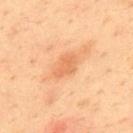image source — total-body-photography crop, ~15 mm field of view
image-analysis metrics — an average lesion color of about L≈61 a*≈26 b*≈39 (CIELAB), about 8 CIELAB-L* units darker than the surrounding skin, and a lesion-to-skin contrast of about 5.5 (normalized; higher = more distinct); a within-lesion color-variation index near 1/10 and a peripheral color-asymmetry measure near 0.5
patient — male, approximately 35 years of age
site — the upper back
lighting — cross-polarized illumination
lesion diameter — ≈3 mm